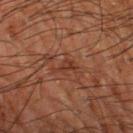follow-up: total-body-photography surveillance lesion; no biopsy
subject: male, roughly 65 years of age
lighting: cross-polarized
site: the left thigh
acquisition: ~15 mm tile from a whole-body skin photo
TBP lesion metrics: an area of roughly 3 mm², an outline eccentricity of about 0.85 (0 = round, 1 = elongated), and a shape-asymmetry score of about 0.5 (0 = symmetric); a lesion color around L≈29 a*≈20 b*≈25 in CIELAB, a lesion–skin lightness drop of about 6, and a lesion-to-skin contrast of about 6.5 (normalized; higher = more distinct)
lesion size: ≈3 mm